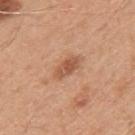Q: Is there a histopathology result?
A: no biopsy performed (imaged during a skin exam)
Q: What did automated image analysis measure?
A: a nevus-likeness score of about 70/100 and lesion-presence confidence of about 100/100
Q: What is the anatomic site?
A: the mid back
Q: How large is the lesion?
A: ≈3.5 mm
Q: What kind of image is this?
A: ~15 mm crop, total-body skin-cancer survey
Q: Patient demographics?
A: male, in their 50s